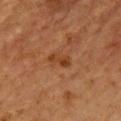| key | value |
|---|---|
| biopsy status | no biopsy performed (imaged during a skin exam) |
| image | total-body-photography crop, ~15 mm field of view |
| illumination | cross-polarized |
| anatomic site | the front of the torso |
| patient | male, aged approximately 60 |